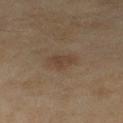Imaged during a routine full-body skin examination; the lesion was not biopsied and no histopathology is available.
The patient is a female in their 60s.
Measured at roughly 3.5 mm in maximum diameter.
The lesion is on the left lower leg.
Imaged with cross-polarized lighting.
Automated image analysis of the tile measured a footprint of about 5.5 mm² and a shape eccentricity near 0.8.
A 15 mm crop from a total-body photograph taken for skin-cancer surveillance.